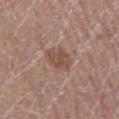This lesion was catalogued during total-body skin photography and was not selected for biopsy.
The lesion-visualizer software estimated an outline eccentricity of about 0.55 (0 = round, 1 = elongated). And it measured an automated nevus-likeness rating near 15 out of 100 and a detector confidence of about 100 out of 100 that the crop contains a lesion.
The subject is a male aged 73–77.
On the left lower leg.
Imaged with white-light lighting.
Cropped from a whole-body photographic skin survey; the tile spans about 15 mm.
About 3 mm across.